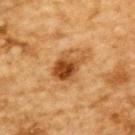Clinical impression:
The lesion was photographed on a routine skin check and not biopsied; there is no pathology result.
Clinical summary:
A male patient, roughly 85 years of age or older. On the upper back. A 15 mm close-up tile from a total-body photography series done for melanoma screening.